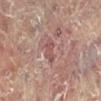Q: Is there a histopathology result?
A: no biopsy performed (imaged during a skin exam)
Q: What are the patient's age and sex?
A: female, aged around 80
Q: Where on the body is the lesion?
A: the left leg
Q: What lighting was used for the tile?
A: cross-polarized
Q: What kind of image is this?
A: 15 mm crop, total-body photography
Q: Automated lesion metrics?
A: a lesion color around L≈46 a*≈22 b*≈20 in CIELAB and roughly 7 lightness units darker than nearby skin
Q: What is the lesion's diameter?
A: ≈3.5 mm